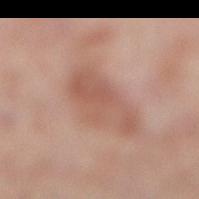From the left lower leg.
Longest diameter approximately 6.5 mm.
A female patient in their 50s.
The tile uses cross-polarized illumination.
A close-up tile cropped from a whole-body skin photograph, about 15 mm across.